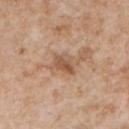Case summary:
* workup — imaged on a skin check; not biopsied
* body site — the chest
* subject — male, aged around 65
* automated lesion analysis — a footprint of about 5 mm², an outline eccentricity of about 0.75 (0 = round, 1 = elongated), and two-axis asymmetry of about 0.3; a border-irregularity rating of about 3/10, a color-variation rating of about 4/10, and radial color variation of about 1.5
* image source — ~15 mm crop, total-body skin-cancer survey
* lesion size — about 3 mm
* lighting — white-light illumination